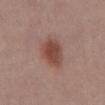Part of a total-body skin-imaging series; this lesion was reviewed on a skin check and was not flagged for biopsy.
The tile uses white-light illumination.
Measured at roughly 4.5 mm in maximum diameter.
A female subject, approximately 40 years of age.
The lesion is on the abdomen.
A lesion tile, about 15 mm wide, cut from a 3D total-body photograph.